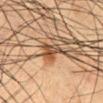Impression:
The lesion was tiled from a total-body skin photograph and was not biopsied.
Acquisition and patient details:
From the abdomen. A male subject aged 48 to 52. A 15 mm close-up tile from a total-body photography series done for melanoma screening.